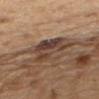The subject is a male aged 68 to 72. The lesion's longest dimension is about 4.5 mm. On the upper back. Cropped from a total-body skin-imaging series; the visible field is about 15 mm. This is a white-light tile.